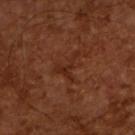Assessment:
The lesion was photographed on a routine skin check and not biopsied; there is no pathology result.
Context:
The subject is a male roughly 65 years of age. A region of skin cropped from a whole-body photographic capture, roughly 15 mm wide. The tile uses cross-polarized illumination. Longest diameter approximately 3.5 mm. The lesion-visualizer software estimated a lesion area of about 4.5 mm², an outline eccentricity of about 0.7 (0 = round, 1 = elongated), and a symmetry-axis asymmetry near 0.75. It also reported a lesion color around L≈26 a*≈23 b*≈28 in CIELAB, a lesion–skin lightness drop of about 5, and a normalized lesion–skin contrast near 5.5. And it measured a nevus-likeness score of about 0/100 and a detector confidence of about 100 out of 100 that the crop contains a lesion.